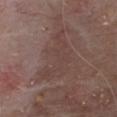<tbp_lesion>
  <site>chest</site>
  <patient>
    <sex>male</sex>
    <age_approx>80</age_approx>
  </patient>
  <image>
    <source>total-body photography crop</source>
    <field_of_view_mm>15</field_of_view_mm>
  </image>
</tbp_lesion>